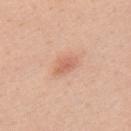Notes:
- biopsy status: imaged on a skin check; not biopsied
- body site: the upper back
- lesion size: about 3 mm
- lighting: white-light illumination
- subject: female, aged 38–42
- acquisition: ~15 mm tile from a whole-body skin photo